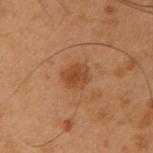Q: Is there a histopathology result?
A: imaged on a skin check; not biopsied
Q: What kind of image is this?
A: ~15 mm tile from a whole-body skin photo
Q: Patient demographics?
A: male, in their mid-50s
Q: What is the anatomic site?
A: the right upper arm